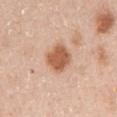<case>
  <biopsy_status>not biopsied; imaged during a skin examination</biopsy_status>
  <lesion_size>
    <long_diameter_mm_approx>3.5</long_diameter_mm_approx>
  </lesion_size>
  <site>left upper arm</site>
  <patient>
    <sex>female</sex>
    <age_approx>50</age_approx>
  </patient>
  <lighting>white-light</lighting>
  <image>
    <source>total-body photography crop</source>
    <field_of_view_mm>15</field_of_view_mm>
  </image>
</case>